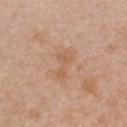Clinical impression:
The lesion was tiled from a total-body skin photograph and was not biopsied.
Acquisition and patient details:
A close-up tile cropped from a whole-body skin photograph, about 15 mm across. An algorithmic analysis of the crop reported a footprint of about 5 mm², an outline eccentricity of about 0.9 (0 = round, 1 = elongated), and a shape-asymmetry score of about 0.4 (0 = symmetric). And it measured internal color variation of about 1.5 on a 0–10 scale. The analysis additionally found a nevus-likeness score of about 0/100. A female subject aged 38 to 42. From the front of the torso. Imaged with white-light lighting. The recorded lesion diameter is about 3.5 mm.